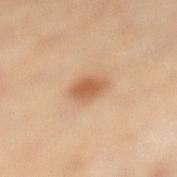Q: Was this lesion biopsied?
A: imaged on a skin check; not biopsied